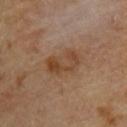Q: Was a biopsy performed?
A: no biopsy performed (imaged during a skin exam)
Q: What kind of image is this?
A: ~15 mm crop, total-body skin-cancer survey
Q: What did automated image analysis measure?
A: an area of roughly 12 mm², an outline eccentricity of about 0.8 (0 = round, 1 = elongated), and two-axis asymmetry of about 0.2; a mean CIELAB color near L≈43 a*≈17 b*≈30, about 7 CIELAB-L* units darker than the surrounding skin, and a normalized border contrast of about 6.5; a within-lesion color-variation index near 5/10 and a peripheral color-asymmetry measure near 1.5; a classifier nevus-likeness of about 0/100 and a detector confidence of about 100 out of 100 that the crop contains a lesion
Q: Lesion size?
A: ~5 mm (longest diameter)
Q: What is the anatomic site?
A: the upper back
Q: What lighting was used for the tile?
A: cross-polarized illumination
Q: Patient demographics?
A: male, aged approximately 85 or older